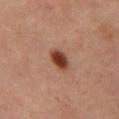The lesion was tiled from a total-body skin photograph and was not biopsied. A lesion tile, about 15 mm wide, cut from a 3D total-body photograph. The patient is a male aged 58–62. On the abdomen.